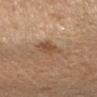<tbp_lesion>
  <biopsy_status>not biopsied; imaged during a skin examination</biopsy_status>
  <image>
    <source>total-body photography crop</source>
    <field_of_view_mm>15</field_of_view_mm>
  </image>
  <patient>
    <sex>female</sex>
    <age_approx>40</age_approx>
  </patient>
  <lesion_size>
    <long_diameter_mm_approx>3.0</long_diameter_mm_approx>
  </lesion_size>
  <site>left arm</site>
</tbp_lesion>